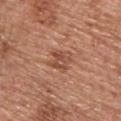Part of a total-body skin-imaging series; this lesion was reviewed on a skin check and was not flagged for biopsy. Cropped from a total-body skin-imaging series; the visible field is about 15 mm. Imaged with white-light lighting. The patient is a male roughly 55 years of age. About 3 mm across. Located on the back.